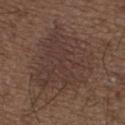Imaged during a routine full-body skin examination; the lesion was not biopsied and no histopathology is available. Approximately 9 mm at its widest. The lesion is located on the upper back. A male patient aged around 50. Cropped from a total-body skin-imaging series; the visible field is about 15 mm. Captured under white-light illumination.